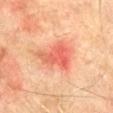{"biopsy_status": "not biopsied; imaged during a skin examination", "automated_metrics": {"area_mm2_approx": 11.0, "eccentricity": 0.6, "shape_asymmetry": 0.45, "border_irregularity_0_10": 4.5, "color_variation_0_10": 6.5, "peripheral_color_asymmetry": 2.5, "nevus_likeness_0_100": 0, "lesion_detection_confidence_0_100": 100}, "patient": {"sex": "male", "age_approx": 75}, "lighting": "cross-polarized", "image": {"source": "total-body photography crop", "field_of_view_mm": 15}, "site": "abdomen"}